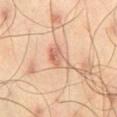Assessment:
This lesion was catalogued during total-body skin photography and was not selected for biopsy.
Acquisition and patient details:
The lesion's longest dimension is about 4 mm. Located on the right thigh. A 15 mm crop from a total-body photograph taken for skin-cancer surveillance. Captured under cross-polarized illumination. A male patient, in their mid- to late 40s.